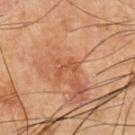- workup: catalogued during a skin exam; not biopsied
- lesion diameter: about 3 mm
- site: the chest
- image source: total-body-photography crop, ~15 mm field of view
- lighting: cross-polarized
- patient: male, aged 63 to 67